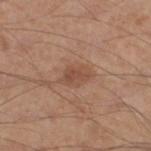The lesion was tiled from a total-body skin photograph and was not biopsied. An algorithmic analysis of the crop reported an area of roughly 5.5 mm² and two-axis asymmetry of about 0.25. And it measured a mean CIELAB color near L≈49 a*≈20 b*≈29, roughly 8 lightness units darker than nearby skin, and a normalized lesion–skin contrast near 6. It also reported a border-irregularity rating of about 2.5/10 and internal color variation of about 3 on a 0–10 scale. On the left lower leg. Approximately 3.5 mm at its widest. A region of skin cropped from a whole-body photographic capture, roughly 15 mm wide. A male subject approximately 55 years of age.